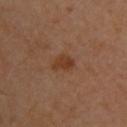Assessment:
This lesion was catalogued during total-body skin photography and was not selected for biopsy.
Background:
This image is a 15 mm lesion crop taken from a total-body photograph. A female patient approximately 40 years of age. The lesion is on the upper back.